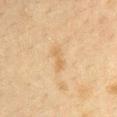Notes:
– workup: imaged on a skin check; not biopsied